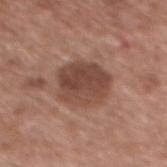biopsy status = total-body-photography surveillance lesion; no biopsy | subject = female, aged 73 to 77 | image source = 15 mm crop, total-body photography | TBP lesion metrics = a lesion area of about 17 mm², a shape eccentricity near 0.6, and a symmetry-axis asymmetry near 0.15; a peripheral color-asymmetry measure near 2; a lesion-detection confidence of about 100/100 | body site = the back.